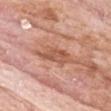| feature | finding |
|---|---|
| biopsy status | no biopsy performed (imaged during a skin exam) |
| imaging modality | ~15 mm crop, total-body skin-cancer survey |
| site | the head or neck |
| patient | male, aged approximately 75 |
| lighting | white-light illumination |
| image-analysis metrics | a footprint of about 8 mm², a shape eccentricity near 0.9, and two-axis asymmetry of about 0.3; an average lesion color of about L≈56 a*≈25 b*≈32 (CIELAB), about 9 CIELAB-L* units darker than the surrounding skin, and a lesion-to-skin contrast of about 6.5 (normalized; higher = more distinct); lesion-presence confidence of about 100/100 |